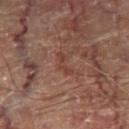From the right thigh.
The subject is a male about 70 years old.
A 15 mm close-up extracted from a 3D total-body photography capture.